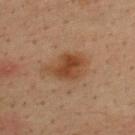Assessment: No biopsy was performed on this lesion — it was imaged during a full skin examination and was not determined to be concerning. Context: The lesion is located on the upper back. Measured at roughly 5 mm in maximum diameter. A male patient, aged approximately 35. Cropped from a whole-body photographic skin survey; the tile spans about 15 mm.